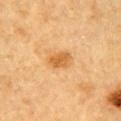Imaged during a routine full-body skin examination; the lesion was not biopsied and no histopathology is available. This is a cross-polarized tile. The recorded lesion diameter is about 3 mm. The total-body-photography lesion software estimated roughly 10 lightness units darker than nearby skin and a lesion-to-skin contrast of about 7.5 (normalized; higher = more distinct). The software also gave a border-irregularity rating of about 2/10 and a color-variation rating of about 2.5/10. The analysis additionally found a classifier nevus-likeness of about 80/100 and lesion-presence confidence of about 100/100. A roughly 15 mm field-of-view crop from a total-body skin photograph. A male patient aged around 85 or older. Located on the arm.